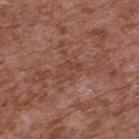Captured during whole-body skin photography for melanoma surveillance; the lesion was not biopsied. The subject is a male in their mid-70s. Located on the upper back. A roughly 15 mm field-of-view crop from a total-body skin photograph.